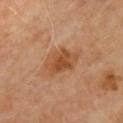Notes:
* biopsy status: total-body-photography surveillance lesion; no biopsy
* illumination: cross-polarized illumination
* acquisition: 15 mm crop, total-body photography
* size: ~4.5 mm (longest diameter)
* anatomic site: the chest
* subject: male, approximately 65 years of age